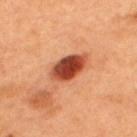The lesion was photographed on a routine skin check and not biopsied; there is no pathology result. A region of skin cropped from a whole-body photographic capture, roughly 15 mm wide. Located on the upper back. About 5 mm across. A male patient, aged 48–52.